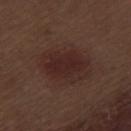Assessment:
This lesion was catalogued during total-body skin photography and was not selected for biopsy.
Acquisition and patient details:
Measured at roughly 5.5 mm in maximum diameter. On the left thigh. Cropped from a total-body skin-imaging series; the visible field is about 15 mm. The subject is a male approximately 70 years of age.